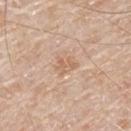biopsy_status: not biopsied; imaged during a skin examination
site: mid back
image:
  source: total-body photography crop
  field_of_view_mm: 15
patient:
  sex: male
  age_approx: 80
lesion_size:
  long_diameter_mm_approx: 2.5
lighting: white-light